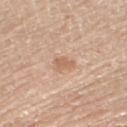Q: Was a biopsy performed?
A: no biopsy performed (imaged during a skin exam)
Q: Patient demographics?
A: female, about 70 years old
Q: What kind of image is this?
A: ~15 mm tile from a whole-body skin photo
Q: Illumination type?
A: white-light illumination
Q: What did automated image analysis measure?
A: a lesion color around L≈62 a*≈19 b*≈32 in CIELAB, about 9 CIELAB-L* units darker than the surrounding skin, and a lesion-to-skin contrast of about 6 (normalized; higher = more distinct); a border-irregularity rating of about 3/10, a color-variation rating of about 1/10, and radial color variation of about 0.5; an automated nevus-likeness rating near 0 out of 100 and a lesion-detection confidence of about 100/100
Q: Lesion location?
A: the leg